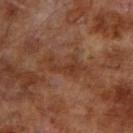follow-up: imaged on a skin check; not biopsied | body site: the right upper arm | image source: 15 mm crop, total-body photography | subject: male, about 65 years old | TBP lesion metrics: an outline eccentricity of about 0.9 (0 = round, 1 = elongated) and two-axis asymmetry of about 0.6; a lesion color around L≈34 a*≈22 b*≈29 in CIELAB and a normalized border contrast of about 6.5.